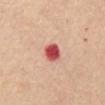Case summary:
* biopsy status — total-body-photography surveillance lesion; no biopsy
* size — ≈2.5 mm
* subject — female, aged 63 to 67
* illumination — white-light
* site — the abdomen
* image-analysis metrics — an automated nevus-likeness rating near 0 out of 100 and a detector confidence of about 100 out of 100 that the crop contains a lesion
* acquisition — ~15 mm tile from a whole-body skin photo